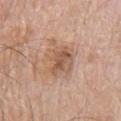Imaged during a routine full-body skin examination; the lesion was not biopsied and no histopathology is available.
Measured at roughly 5 mm in maximum diameter.
The tile uses white-light illumination.
An algorithmic analysis of the crop reported an eccentricity of roughly 0.75 and two-axis asymmetry of about 0.4. The analysis additionally found a border-irregularity index near 6/10, a color-variation rating of about 5.5/10, and peripheral color asymmetry of about 2. And it measured a classifier nevus-likeness of about 0/100.
The patient is a male aged approximately 80.
A 15 mm crop from a total-body photograph taken for skin-cancer surveillance.
The lesion is on the chest.